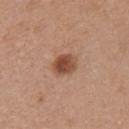The lesion was tiled from a total-body skin photograph and was not biopsied.
A female patient, aged 33 to 37.
This image is a 15 mm lesion crop taken from a total-body photograph.
About 3 mm across.
Captured under white-light illumination.
An algorithmic analysis of the crop reported an automated nevus-likeness rating near 95 out of 100 and a detector confidence of about 100 out of 100 that the crop contains a lesion.
Located on the right upper arm.